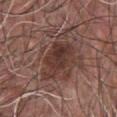Q: Was this lesion biopsied?
A: total-body-photography surveillance lesion; no biopsy
Q: Lesion location?
A: the chest
Q: What kind of image is this?
A: ~15 mm crop, total-body skin-cancer survey
Q: Lesion size?
A: about 6 mm
Q: What are the patient's age and sex?
A: male, aged around 50